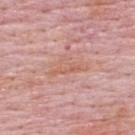{"biopsy_status": "not biopsied; imaged during a skin examination", "patient": {"sex": "male", "age_approx": 65}, "image": {"source": "total-body photography crop", "field_of_view_mm": 15}, "lesion_size": {"long_diameter_mm_approx": 4.5}, "lighting": "white-light", "automated_metrics": {"nevus_likeness_0_100": 0, "lesion_detection_confidence_0_100": 55}, "site": "upper back"}